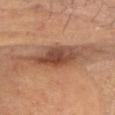Q: Was this lesion biopsied?
A: no biopsy performed (imaged during a skin exam)
Q: Automated lesion metrics?
A: a normalized lesion–skin contrast near 10.5; an automated nevus-likeness rating near 90 out of 100 and a detector confidence of about 100 out of 100 that the crop contains a lesion
Q: Who is the patient?
A: male, aged approximately 70
Q: What is the imaging modality?
A: total-body-photography crop, ~15 mm field of view
Q: How large is the lesion?
A: about 5.5 mm
Q: What lighting was used for the tile?
A: white-light illumination
Q: Lesion location?
A: the head or neck